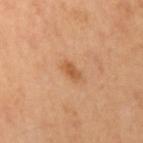Impression:
The lesion was photographed on a routine skin check and not biopsied; there is no pathology result.
Background:
A lesion tile, about 15 mm wide, cut from a 3D total-body photograph. Automated tile analysis of the lesion measured an area of roughly 3 mm² and an eccentricity of roughly 0.8. The analysis additionally found a classifier nevus-likeness of about 75/100. The tile uses cross-polarized illumination. The lesion is on the left arm. A female patient aged 58 to 62. Longest diameter approximately 2.5 mm.